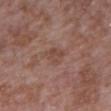Captured during whole-body skin photography for melanoma surveillance; the lesion was not biopsied. Automated image analysis of the tile measured a lesion color around L≈45 a*≈19 b*≈24 in CIELAB, a lesion–skin lightness drop of about 7, and a lesion-to-skin contrast of about 5.5 (normalized; higher = more distinct). Located on the right upper arm. A roughly 15 mm field-of-view crop from a total-body skin photograph. A female patient, approximately 70 years of age. About 3 mm across. This is a white-light tile.